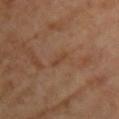follow-up=no biopsy performed (imaged during a skin exam) | lighting=cross-polarized | imaging modality=~15 mm crop, total-body skin-cancer survey | lesion size=~2.5 mm (longest diameter) | patient=female, in their mid-60s | site=the front of the torso.